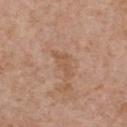• biopsy status · catalogued during a skin exam; not biopsied
• imaging modality · 15 mm crop, total-body photography
• anatomic site · the front of the torso
• subject · female, aged approximately 65
• lesion diameter · ≈3.5 mm
• tile lighting · white-light illumination